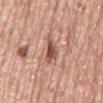Findings:
• workup — imaged on a skin check; not biopsied